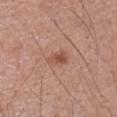Q: Is there a histopathology result?
A: total-body-photography surveillance lesion; no biopsy
Q: Lesion location?
A: the right upper arm
Q: Lesion size?
A: ~2.5 mm (longest diameter)
Q: Patient demographics?
A: male, aged 53–57
Q: What kind of image is this?
A: ~15 mm crop, total-body skin-cancer survey